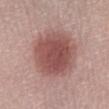Assessment: No biopsy was performed on this lesion — it was imaged during a full skin examination and was not determined to be concerning. Acquisition and patient details: Imaged with white-light lighting. A female patient about 30 years old. Approximately 6 mm at its widest. An algorithmic analysis of the crop reported border irregularity of about 1.5 on a 0–10 scale and a peripheral color-asymmetry measure near 1. The analysis additionally found a classifier nevus-likeness of about 100/100 and a detector confidence of about 100 out of 100 that the crop contains a lesion. A 15 mm close-up tile from a total-body photography series done for melanoma screening. From the left lower leg.